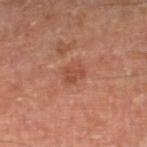notes — no biopsy performed (imaged during a skin exam) | automated lesion analysis — a mean CIELAB color near L≈47 a*≈26 b*≈31 and a normalized lesion–skin contrast near 5.5; a detector confidence of about 100 out of 100 that the crop contains a lesion | image — ~15 mm tile from a whole-body skin photo | tile lighting — cross-polarized illumination | patient — male, roughly 70 years of age | diameter — about 2.5 mm | location — the right lower leg.